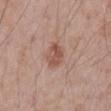Q: Was a biopsy performed?
A: imaged on a skin check; not biopsied
Q: How large is the lesion?
A: ~3.5 mm (longest diameter)
Q: What is the anatomic site?
A: the abdomen
Q: Illumination type?
A: white-light illumination
Q: What are the patient's age and sex?
A: male, approximately 70 years of age
Q: What kind of image is this?
A: total-body-photography crop, ~15 mm field of view